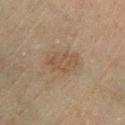The lesion was tiled from a total-body skin photograph and was not biopsied. Located on the left lower leg. A female subject aged 53–57. Imaged with cross-polarized lighting. A 15 mm close-up extracted from a 3D total-body photography capture. About 4 mm across. An algorithmic analysis of the crop reported a lesion–skin lightness drop of about 6 and a normalized border contrast of about 5.5. The analysis additionally found a border-irregularity rating of about 2.5/10, internal color variation of about 2.5 on a 0–10 scale, and a peripheral color-asymmetry measure near 1. It also reported a classifier nevus-likeness of about 25/100.